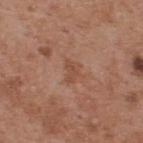workup=total-body-photography surveillance lesion; no biopsy
subject=male, approximately 55 years of age
imaging modality=~15 mm tile from a whole-body skin photo
site=the upper back
illumination=white-light illumination